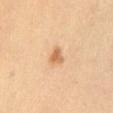Part of a total-body skin-imaging series; this lesion was reviewed on a skin check and was not flagged for biopsy. The tile uses cross-polarized illumination. A lesion tile, about 15 mm wide, cut from a 3D total-body photograph. The lesion is on the abdomen. A male patient, about 55 years old.